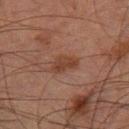biopsy status — no biopsy performed (imaged during a skin exam)
imaging modality — total-body-photography crop, ~15 mm field of view
location — the right thigh
patient — male, approximately 70 years of age